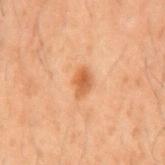lighting=cross-polarized | body site=the back | subject=male, about 50 years old | lesion size=~3 mm (longest diameter) | automated metrics=a mean CIELAB color near L≈46 a*≈21 b*≈33 and a normalized lesion–skin contrast near 7.5 | imaging modality=~15 mm tile from a whole-body skin photo.